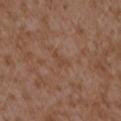Findings:
• workup: catalogued during a skin exam; not biopsied
• image: 15 mm crop, total-body photography
• location: the left forearm
• subject: female, aged 33 to 37